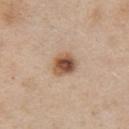biopsy_status: not biopsied; imaged during a skin examination
image:
  source: total-body photography crop
  field_of_view_mm: 15
patient:
  sex: female
  age_approx: 45
site: chest